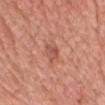Q: Was this lesion biopsied?
A: imaged on a skin check; not biopsied
Q: Who is the patient?
A: male, roughly 55 years of age
Q: What kind of image is this?
A: ~15 mm tile from a whole-body skin photo
Q: What did automated image analysis measure?
A: an average lesion color of about L≈54 a*≈27 b*≈29 (CIELAB) and roughly 8 lightness units darker than nearby skin; a classifier nevus-likeness of about 0/100 and a detector confidence of about 100 out of 100 that the crop contains a lesion
Q: What is the anatomic site?
A: the head or neck
Q: Lesion size?
A: ≈2.5 mm
Q: Illumination type?
A: white-light illumination